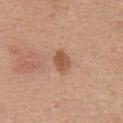subject: female, roughly 40 years of age | lesion diameter: ~3 mm (longest diameter) | image: total-body-photography crop, ~15 mm field of view | anatomic site: the upper back.